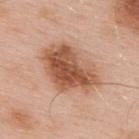From the upper back. A 15 mm crop from a total-body photograph taken for skin-cancer surveillance. A male subject, aged around 55.